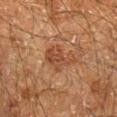Imaged during a routine full-body skin examination; the lesion was not biopsied and no histopathology is available. A lesion tile, about 15 mm wide, cut from a 3D total-body photograph. Automated image analysis of the tile measured an automated nevus-likeness rating near 20 out of 100 and a lesion-detection confidence of about 100/100. On the right lower leg. The patient is a male aged around 60.